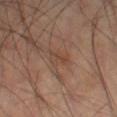Recorded during total-body skin imaging; not selected for excision or biopsy.
This image is a 15 mm lesion crop taken from a total-body photograph.
Captured under cross-polarized illumination.
An algorithmic analysis of the crop reported a footprint of about 4 mm² and an outline eccentricity of about 0.8 (0 = round, 1 = elongated). The analysis additionally found a lesion color around L≈42 a*≈18 b*≈27 in CIELAB, a lesion–skin lightness drop of about 5, and a normalized border contrast of about 5. It also reported border irregularity of about 3 on a 0–10 scale, a color-variation rating of about 3/10, and radial color variation of about 1. It also reported an automated nevus-likeness rating near 0 out of 100 and a detector confidence of about 100 out of 100 that the crop contains a lesion.
Located on the left thigh.
Measured at roughly 2.5 mm in maximum diameter.
The subject is a male in their 60s.